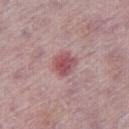biopsy status — total-body-photography surveillance lesion; no biopsy
imaging modality — total-body-photography crop, ~15 mm field of view
site — the right thigh
patient — male, roughly 75 years of age